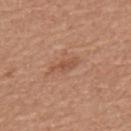Recorded during total-body skin imaging; not selected for excision or biopsy.
Imaged with white-light lighting.
The subject is a male in their mid- to late 60s.
About 3.5 mm across.
A close-up tile cropped from a whole-body skin photograph, about 15 mm across.
On the mid back.